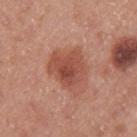A female patient aged 38 to 42.
A roughly 15 mm field-of-view crop from a total-body skin photograph.
Located on the arm.
An algorithmic analysis of the crop reported a lesion area of about 14 mm², a shape eccentricity near 0.45, and two-axis asymmetry of about 0.3. The analysis additionally found a classifier nevus-likeness of about 85/100.